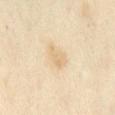| field | value |
|---|---|
| notes | no biopsy performed (imaged during a skin exam) |
| lesion diameter | ≈3.5 mm |
| subject | female, aged 33 to 37 |
| location | the mid back |
| illumination | cross-polarized illumination |
| automated metrics | an outline eccentricity of about 0.9 (0 = round, 1 = elongated); a mean CIELAB color near L≈72 a*≈12 b*≈36, a lesion–skin lightness drop of about 7, and a normalized lesion–skin contrast near 5; a border-irregularity rating of about 4.5/10, a within-lesion color-variation index near 1.5/10, and peripheral color asymmetry of about 0.5; an automated nevus-likeness rating near 5 out of 100 and a detector confidence of about 100 out of 100 that the crop contains a lesion |
| image | 15 mm crop, total-body photography |